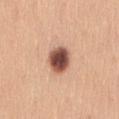Q: Lesion location?
A: the abdomen
Q: How was the tile lit?
A: white-light
Q: Patient demographics?
A: female, aged around 25
Q: How large is the lesion?
A: ≈3.5 mm
Q: How was this image acquired?
A: 15 mm crop, total-body photography